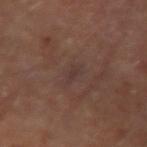Assessment: The lesion was photographed on a routine skin check and not biopsied; there is no pathology result. Background: The lesion is on the right upper arm. Measured at roughly 2.5 mm in maximum diameter. A roughly 15 mm field-of-view crop from a total-body skin photograph. The patient is a male aged 63 to 67. The lesion-visualizer software estimated an average lesion color of about L≈33 a*≈15 b*≈18 (CIELAB), a lesion–skin lightness drop of about 4, and a lesion-to-skin contrast of about 5.5 (normalized; higher = more distinct).